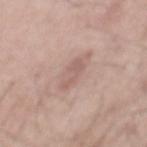  biopsy_status: not biopsied; imaged during a skin examination
  patient:
    sex: male
    age_approx: 55
  image:
    source: total-body photography crop
    field_of_view_mm: 15
  site: mid back